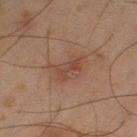Clinical summary: This is a cross-polarized tile. From the leg. The lesion's longest dimension is about 3 mm. A male patient, aged 43–47. A region of skin cropped from a whole-body photographic capture, roughly 15 mm wide.